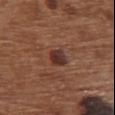• biopsy status — catalogued during a skin exam; not biopsied
• image — ~15 mm tile from a whole-body skin photo
• illumination — white-light illumination
• diameter — ≈2.5 mm
• location — the chest
• subject — female, in their mid- to late 70s